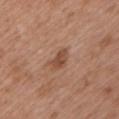The lesion was photographed on a routine skin check and not biopsied; there is no pathology result. Longest diameter approximately 3 mm. A male subject, in their mid-50s. Cropped from a whole-body photographic skin survey; the tile spans about 15 mm. The lesion is on the left upper arm. The tile uses white-light illumination.